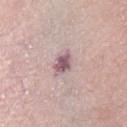Assessment:
Captured during whole-body skin photography for melanoma surveillance; the lesion was not biopsied.
Acquisition and patient details:
The lesion is located on the front of the torso. Longest diameter approximately 3 mm. A region of skin cropped from a whole-body photographic capture, roughly 15 mm wide. This is a white-light tile. An algorithmic analysis of the crop reported an eccentricity of roughly 0.7 and two-axis asymmetry of about 0.3. The analysis additionally found an automated nevus-likeness rating near 0 out of 100 and a detector confidence of about 95 out of 100 that the crop contains a lesion. The subject is a female in their mid- to late 70s.